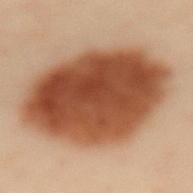This lesion was catalogued during total-body skin photography and was not selected for biopsy. Longest diameter approximately 12 mm. A female patient about 60 years old. The tile uses cross-polarized illumination. The total-body-photography lesion software estimated an average lesion color of about L≈42 a*≈22 b*≈30 (CIELAB), roughly 17 lightness units darker than nearby skin, and a normalized lesion–skin contrast near 13. The software also gave a border-irregularity rating of about 1.5/10 and a within-lesion color-variation index near 6.5/10. And it measured a classifier nevus-likeness of about 100/100 and a lesion-detection confidence of about 100/100. A 15 mm crop from a total-body photograph taken for skin-cancer surveillance. From the mid back.